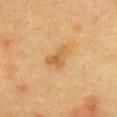Q: Was a biopsy performed?
A: total-body-photography surveillance lesion; no biopsy
Q: What lighting was used for the tile?
A: cross-polarized illumination
Q: Lesion location?
A: the abdomen
Q: What is the imaging modality?
A: total-body-photography crop, ~15 mm field of view
Q: What are the patient's age and sex?
A: female, in their mid-50s